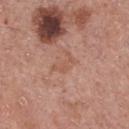Impression:
No biopsy was performed on this lesion — it was imaged during a full skin examination and was not determined to be concerning.
Context:
A lesion tile, about 15 mm wide, cut from a 3D total-body photograph. The patient is a male approximately 70 years of age. From the chest. Imaged with white-light lighting. Automated tile analysis of the lesion measured a shape eccentricity near 0.85 and a symmetry-axis asymmetry near 0.65.